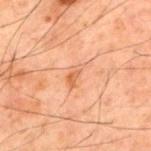This lesion was catalogued during total-body skin photography and was not selected for biopsy. This is a cross-polarized tile. A region of skin cropped from a whole-body photographic capture, roughly 15 mm wide. Located on the back. Automated tile analysis of the lesion measured roughly 6 lightness units darker than nearby skin. A male subject, about 60 years old.